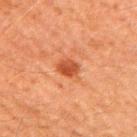Imaged during a routine full-body skin examination; the lesion was not biopsied and no histopathology is available. Located on the arm. The lesion-visualizer software estimated a footprint of about 4.5 mm², an eccentricity of roughly 0.6, and a shape-asymmetry score of about 0.2 (0 = symmetric). The analysis additionally found an average lesion color of about L≈40 a*≈27 b*≈34 (CIELAB), roughly 10 lightness units darker than nearby skin, and a normalized border contrast of about 8. This is a cross-polarized tile. A male patient aged around 60. A 15 mm close-up tile from a total-body photography series done for melanoma screening.